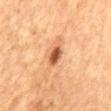Assessment: The lesion was tiled from a total-body skin photograph and was not biopsied. Image and clinical context: The lesion is located on the mid back. A close-up tile cropped from a whole-body skin photograph, about 15 mm across. A female patient, about 60 years old. Automated image analysis of the tile measured a lesion color around L≈52 a*≈27 b*≈38 in CIELAB, a lesion–skin lightness drop of about 16, and a normalized lesion–skin contrast near 10.5. The analysis additionally found radial color variation of about 1. The analysis additionally found an automated nevus-likeness rating near 90 out of 100 and a lesion-detection confidence of about 100/100. Captured under cross-polarized illumination. Approximately 2.5 mm at its widest.